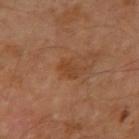No biopsy was performed on this lesion — it was imaged during a full skin examination and was not determined to be concerning.
A male patient, aged 58 to 62.
A region of skin cropped from a whole-body photographic capture, roughly 15 mm wide.
The lesion is located on the right upper arm.